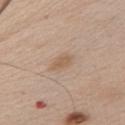Impression: The lesion was photographed on a routine skin check and not biopsied; there is no pathology result. Clinical summary: From the chest. This image is a 15 mm lesion crop taken from a total-body photograph. A male patient, roughly 65 years of age. Automated tile analysis of the lesion measured a lesion color around L≈58 a*≈17 b*≈31 in CIELAB, roughly 8 lightness units darker than nearby skin, and a normalized border contrast of about 6. It also reported border irregularity of about 2.5 on a 0–10 scale, a color-variation rating of about 1/10, and a peripheral color-asymmetry measure near 0. The tile uses white-light illumination.